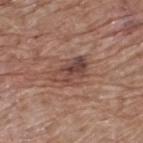notes = catalogued during a skin exam; not biopsied | image source = ~15 mm crop, total-body skin-cancer survey | patient = male, aged 68 to 72 | anatomic site = the upper back.